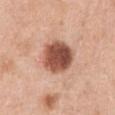Imaged during a routine full-body skin examination; the lesion was not biopsied and no histopathology is available. Imaged with white-light lighting. The lesion is on the chest. A female patient about 40 years old. A 15 mm crop from a total-body photograph taken for skin-cancer surveillance. Approximately 4.5 mm at its widest.